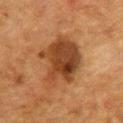Findings:
* notes: total-body-photography surveillance lesion; no biopsy
* tile lighting: cross-polarized
* lesion size: about 6.5 mm
* imaging modality: ~15 mm tile from a whole-body skin photo
* automated metrics: a lesion area of about 23 mm², a shape eccentricity near 0.55, and a shape-asymmetry score of about 0.35 (0 = symmetric); a mean CIELAB color near L≈35 a*≈20 b*≈31 and about 11 CIELAB-L* units darker than the surrounding skin
* patient: female, in their mid- to late 50s
* location: the upper back